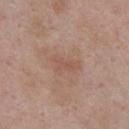Clinical impression:
The lesion was tiled from a total-body skin photograph and was not biopsied.
Clinical summary:
The tile uses white-light illumination. The lesion is located on the chest. Cropped from a total-body skin-imaging series; the visible field is about 15 mm. About 3.5 mm across. The patient is a male about 55 years old.